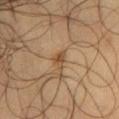Captured during whole-body skin photography for melanoma surveillance; the lesion was not biopsied.
Imaged with cross-polarized lighting.
A region of skin cropped from a whole-body photographic capture, roughly 15 mm wide.
The lesion is located on the chest.
A male patient, aged 58–62.
The lesion's longest dimension is about 3 mm.